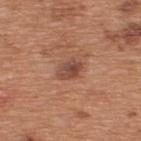Findings:
* workup · imaged on a skin check; not biopsied